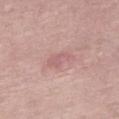Q: Was a biopsy performed?
A: catalogued during a skin exam; not biopsied
Q: Lesion location?
A: the right thigh
Q: What are the patient's age and sex?
A: male, in their 60s
Q: What kind of image is this?
A: 15 mm crop, total-body photography